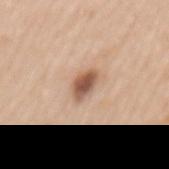follow-up = imaged on a skin check; not biopsied
image = ~15 mm tile from a whole-body skin photo
location = the mid back
patient = female, roughly 70 years of age